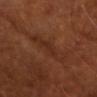{"lighting": "cross-polarized", "site": "chest", "lesion_size": {"long_diameter_mm_approx": 3.0}, "patient": {"sex": "male", "age_approx": 65}, "image": {"source": "total-body photography crop", "field_of_view_mm": 15}}